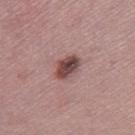workup — catalogued during a skin exam; not biopsied
diameter — ~3.5 mm (longest diameter)
illumination — white-light
location — the right thigh
subject — female, in their mid-40s
image — total-body-photography crop, ~15 mm field of view